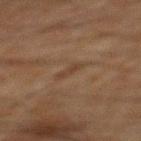<tbp_lesion>
<biopsy_status>not biopsied; imaged during a skin examination</biopsy_status>
<image>
  <source>total-body photography crop</source>
  <field_of_view_mm>15</field_of_view_mm>
</image>
<patient>
  <sex>male</sex>
  <age_approx>65</age_approx>
</patient>
<site>mid back</site>
</tbp_lesion>